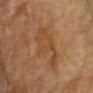This lesion was catalogued during total-body skin photography and was not selected for biopsy.
An algorithmic analysis of the crop reported a lesion area of about 16 mm², an outline eccentricity of about 0.9 (0 = round, 1 = elongated), and a shape-asymmetry score of about 0.35 (0 = symmetric). The analysis additionally found a lesion color around L≈47 a*≈21 b*≈35 in CIELAB, about 6 CIELAB-L* units darker than the surrounding skin, and a normalized lesion–skin contrast near 5. And it measured border irregularity of about 5.5 on a 0–10 scale and radial color variation of about 0.5. And it measured a classifier nevus-likeness of about 0/100 and a lesion-detection confidence of about 100/100.
The tile uses cross-polarized illumination.
Measured at roughly 7 mm in maximum diameter.
A female patient in their mid-70s.
Located on the arm.
A 15 mm close-up tile from a total-body photography series done for melanoma screening.